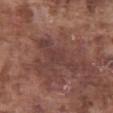Clinical impression: This lesion was catalogued during total-body skin photography and was not selected for biopsy. Background: The recorded lesion diameter is about 6.5 mm. A male patient in their mid-70s. Cropped from a whole-body photographic skin survey; the tile spans about 15 mm. An algorithmic analysis of the crop reported a lesion area of about 10 mm², an outline eccentricity of about 0.95 (0 = round, 1 = elongated), and two-axis asymmetry of about 0.45. And it measured a classifier nevus-likeness of about 0/100. On the abdomen. This is a white-light tile.